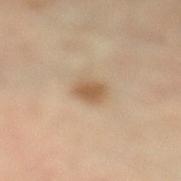<tbp_lesion>
<biopsy_status>not biopsied; imaged during a skin examination</biopsy_status>
<lighting>cross-polarized</lighting>
<automated_metrics>
  <area_mm2_approx>5.5</area_mm2_approx>
  <eccentricity>0.55</eccentricity>
  <shape_asymmetry>0.2</shape_asymmetry>
  <border_irregularity_0_10>1.5</border_irregularity_0_10>
  <color_variation_0_10>2.0</color_variation_0_10>
  <peripheral_color_asymmetry>0.5</peripheral_color_asymmetry>
</automated_metrics>
<site>leg</site>
<image>
  <source>total-body photography crop</source>
  <field_of_view_mm>15</field_of_view_mm>
</image>
<lesion_size>
  <long_diameter_mm_approx>3.0</long_diameter_mm_approx>
</lesion_size>
<patient>
  <sex>female</sex>
  <age_approx>65</age_approx>
</patient>
</tbp_lesion>